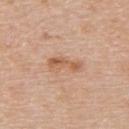follow-up: total-body-photography surveillance lesion; no biopsy | lighting: white-light | subject: male, aged approximately 60 | automated lesion analysis: a lesion area of about 5.5 mm², a shape eccentricity near 0.95, and two-axis asymmetry of about 0.4; a mean CIELAB color near L≈59 a*≈21 b*≈34, a lesion–skin lightness drop of about 9, and a lesion-to-skin contrast of about 7 (normalized; higher = more distinct); border irregularity of about 5.5 on a 0–10 scale | acquisition: 15 mm crop, total-body photography | lesion diameter: ≈4 mm | body site: the back.